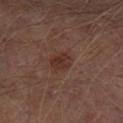biopsy_status: not biopsied; imaged during a skin examination
site: leg
automated_metrics:
  area_mm2_approx: 4.5
  eccentricity: 0.6
  shape_asymmetry: 0.25
  nevus_likeness_0_100: 60
  lesion_detection_confidence_0_100: 100
image:
  source: total-body photography crop
  field_of_view_mm: 15
patient:
  sex: male
  age_approx: 65
lesion_size:
  long_diameter_mm_approx: 3.0
lighting: cross-polarized